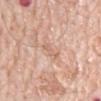Clinical impression:
Imaged during a routine full-body skin examination; the lesion was not biopsied and no histopathology is available.
Background:
A male patient aged around 80. A 15 mm crop from a total-body photograph taken for skin-cancer surveillance. Measured at roughly 3 mm in maximum diameter. Captured under white-light illumination. The lesion is on the mid back.